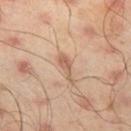Impression:
No biopsy was performed on this lesion — it was imaged during a full skin examination and was not determined to be concerning.
Background:
The lesion is on the leg. A close-up tile cropped from a whole-body skin photograph, about 15 mm across. The patient is a male roughly 45 years of age.